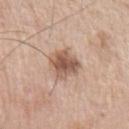  biopsy_status: not biopsied; imaged during a skin examination
  site: left upper arm
  image:
    source: total-body photography crop
    field_of_view_mm: 15
  lesion_size:
    long_diameter_mm_approx: 4.0
  automated_metrics:
    vs_skin_darker_L: 14.0
    vs_skin_contrast_norm: 9.0
    border_irregularity_0_10: 2.5
    color_variation_0_10: 4.5
    peripheral_color_asymmetry: 1.5
    nevus_likeness_0_100: 65
  patient:
    sex: male
    age_approx: 75